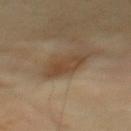{
  "biopsy_status": "not biopsied; imaged during a skin examination",
  "patient": {
    "sex": "male",
    "age_approx": 40
  },
  "site": "upper back",
  "automated_metrics": {
    "lesion_detection_confidence_0_100": 100
  },
  "lesion_size": {
    "long_diameter_mm_approx": 4.0
  },
  "image": {
    "source": "total-body photography crop",
    "field_of_view_mm": 15
  }
}